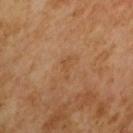No biopsy was performed on this lesion — it was imaged during a full skin examination and was not determined to be concerning.
The recorded lesion diameter is about 2.5 mm.
A male patient, aged 63 to 67.
A lesion tile, about 15 mm wide, cut from a 3D total-body photograph.
The total-body-photography lesion software estimated a mean CIELAB color near L≈48 a*≈20 b*≈35, about 5 CIELAB-L* units darker than the surrounding skin, and a normalized border contrast of about 4.5. The software also gave a border-irregularity index near 5.5/10, a within-lesion color-variation index near 0/10, and radial color variation of about 0.